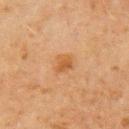The lesion was tiled from a total-body skin photograph and was not biopsied.
Located on the arm.
A roughly 15 mm field-of-view crop from a total-body skin photograph.
Imaged with cross-polarized lighting.
The patient is a male aged approximately 70.
Measured at roughly 3 mm in maximum diameter.
Automated tile analysis of the lesion measured an area of roughly 4.5 mm², a shape eccentricity near 0.65, and a shape-asymmetry score of about 0.35 (0 = symmetric). The software also gave roughly 7 lightness units darker than nearby skin.